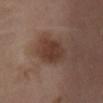• workup: total-body-photography surveillance lesion; no biopsy
• lesion diameter: ≈5 mm
• body site: the chest
• patient: female, aged 53 to 57
• image source: ~15 mm crop, total-body skin-cancer survey
• lighting: white-light